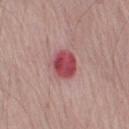| key | value |
|---|---|
| workup | catalogued during a skin exam; not biopsied |
| illumination | white-light illumination |
| body site | the right upper arm |
| TBP lesion metrics | an area of roughly 8 mm², an outline eccentricity of about 0.5 (0 = round, 1 = elongated), and a shape-asymmetry score of about 0.15 (0 = symmetric); a border-irregularity index near 1/10, internal color variation of about 4 on a 0–10 scale, and a peripheral color-asymmetry measure near 1.5; a lesion-detection confidence of about 100/100 |
| acquisition | 15 mm crop, total-body photography |
| patient | male, approximately 70 years of age |
| diameter | ~3.5 mm (longest diameter) |